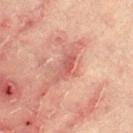notes = no biopsy performed (imaged during a skin exam) | acquisition = total-body-photography crop, ~15 mm field of view | tile lighting = cross-polarized illumination | patient = male, about 65 years old | site = the left thigh.